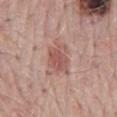This lesion was catalogued during total-body skin photography and was not selected for biopsy. Automated image analysis of the tile measured an eccentricity of roughly 0.55 and two-axis asymmetry of about 0.3. It also reported a lesion color around L≈54 a*≈23 b*≈24 in CIELAB, about 9 CIELAB-L* units darker than the surrounding skin, and a lesion-to-skin contrast of about 6.5 (normalized; higher = more distinct). The analysis additionally found a classifier nevus-likeness of about 70/100. The patient is a male in their 70s. This image is a 15 mm lesion crop taken from a total-body photograph. Approximately 3.5 mm at its widest. This is a white-light tile. The lesion is on the mid back.